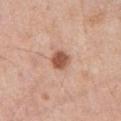biopsy_status: not biopsied; imaged during a skin examination
lighting: white-light
automated_metrics:
  cielab_L: 55
  cielab_a: 23
  cielab_b: 31
  nevus_likeness_0_100: 95
  lesion_detection_confidence_0_100: 100
image:
  source: total-body photography crop
  field_of_view_mm: 15
patient:
  sex: male
  age_approx: 70
lesion_size:
  long_diameter_mm_approx: 2.5
site: abdomen